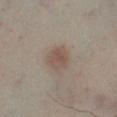No biopsy was performed on this lesion — it was imaged during a full skin examination and was not determined to be concerning. The recorded lesion diameter is about 3 mm. Imaged with cross-polarized lighting. Automated image analysis of the tile measured a lesion area of about 5.5 mm², an eccentricity of roughly 0.5, and two-axis asymmetry of about 0.15. The subject is a male aged around 40. Cropped from a whole-body photographic skin survey; the tile spans about 15 mm. The lesion is located on the left lower leg.